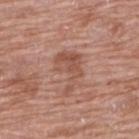notes = imaged on a skin check; not biopsied
body site = the back
automated lesion analysis = a mean CIELAB color near L≈52 a*≈22 b*≈28, roughly 8 lightness units darker than nearby skin, and a normalized border contrast of about 6; a nevus-likeness score of about 0/100 and a detector confidence of about 100 out of 100 that the crop contains a lesion
diameter = about 5 mm
acquisition = ~15 mm crop, total-body skin-cancer survey
subject = female, aged 58 to 62